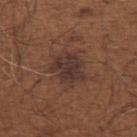follow-up: total-body-photography surveillance lesion; no biopsy | anatomic site: the arm | tile lighting: white-light illumination | image: 15 mm crop, total-body photography | diameter: about 4 mm | patient: male, about 65 years old.